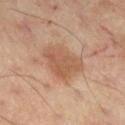Clinical impression: This lesion was catalogued during total-body skin photography and was not selected for biopsy. Context: Automated tile analysis of the lesion measured an area of roughly 14 mm² and a shape-asymmetry score of about 0.3 (0 = symmetric). And it measured an average lesion color of about L≈52 a*≈19 b*≈30 (CIELAB) and a normalized lesion–skin contrast near 6.5. The tile uses cross-polarized illumination. A male subject aged 58 to 62. The lesion is on the right thigh. The recorded lesion diameter is about 5 mm. A region of skin cropped from a whole-body photographic capture, roughly 15 mm wide.